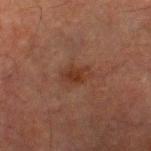Background: A male subject, in their mid- to late 60s. The total-body-photography lesion software estimated internal color variation of about 2 on a 0–10 scale and peripheral color asymmetry of about 0.5. The analysis additionally found lesion-presence confidence of about 100/100. Located on the left lower leg. This is a cross-polarized tile. A close-up tile cropped from a whole-body skin photograph, about 15 mm across.